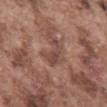Impression:
Imaged during a routine full-body skin examination; the lesion was not biopsied and no histopathology is available.
Clinical summary:
The lesion is located on the back. Cropped from a whole-body photographic skin survey; the tile spans about 15 mm. About 4 mm across. A male subject, aged 73 to 77. The tile uses white-light illumination. Automated image analysis of the tile measured roughly 8 lightness units darker than nearby skin and a normalized lesion–skin contrast near 6.5. And it measured a border-irregularity rating of about 6.5/10 and internal color variation of about 2.5 on a 0–10 scale.